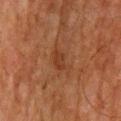Q: Was this lesion biopsied?
A: catalogued during a skin exam; not biopsied
Q: What are the patient's age and sex?
A: male, aged around 80
Q: Where on the body is the lesion?
A: the mid back
Q: Lesion size?
A: about 3.5 mm
Q: What kind of image is this?
A: ~15 mm crop, total-body skin-cancer survey